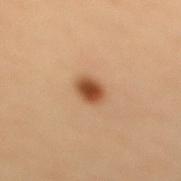Notes:
– biopsy status — total-body-photography surveillance lesion; no biopsy
– location — the upper back
– subject — female, aged around 50
– image source — total-body-photography crop, ~15 mm field of view